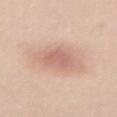notes: total-body-photography surveillance lesion; no biopsy
anatomic site: the abdomen
image: total-body-photography crop, ~15 mm field of view
subject: female, in their mid- to late 40s
TBP lesion metrics: an area of roughly 5.5 mm² and an eccentricity of roughly 0.5; about 8 CIELAB-L* units darker than the surrounding skin and a normalized border contrast of about 5; a within-lesion color-variation index near 1.5/10 and radial color variation of about 0.5
lesion diameter: ≈3 mm